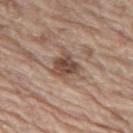Assessment:
Recorded during total-body skin imaging; not selected for excision or biopsy.
Context:
A male patient about 70 years old. The lesion is located on the left thigh. Captured under white-light illumination. About 4.5 mm across. Automated tile analysis of the lesion measured a footprint of about 10 mm², an eccentricity of roughly 0.6, and two-axis asymmetry of about 0.35. The software also gave a border-irregularity rating of about 4.5/10 and a peripheral color-asymmetry measure near 2. A 15 mm crop from a total-body photograph taken for skin-cancer surveillance.